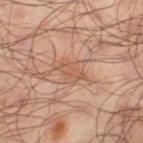biopsy status = imaged on a skin check; not biopsied | patient = male, aged around 65 | imaging modality = 15 mm crop, total-body photography | lighting = cross-polarized illumination | anatomic site = the leg | diameter = ≈3 mm.